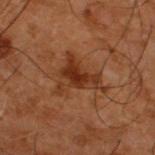Q: Was a biopsy performed?
A: catalogued during a skin exam; not biopsied
Q: How was the tile lit?
A: cross-polarized illumination
Q: Lesion location?
A: the upper back
Q: What kind of image is this?
A: 15 mm crop, total-body photography
Q: What are the patient's age and sex?
A: male, aged around 50